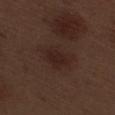The recorded lesion diameter is about 4.5 mm.
Captured under white-light illumination.
Located on the right thigh.
A 15 mm close-up extracted from a 3D total-body photography capture.
A male patient, aged 68–72.
Automated image analysis of the tile measured a color-variation rating of about 2.5/10 and peripheral color asymmetry of about 1.